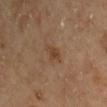Q: Was a biopsy performed?
A: imaged on a skin check; not biopsied
Q: What is the imaging modality?
A: ~15 mm tile from a whole-body skin photo
Q: Automated lesion metrics?
A: an average lesion color of about L≈40 a*≈17 b*≈30 (CIELAB), a lesion–skin lightness drop of about 7, and a lesion-to-skin contrast of about 6.5 (normalized; higher = more distinct); a classifier nevus-likeness of about 5/100 and lesion-presence confidence of about 100/100
Q: Who is the patient?
A: male, aged approximately 65
Q: What is the anatomic site?
A: the upper back
Q: What is the lesion's diameter?
A: ≈2.5 mm